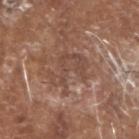- follow-up: total-body-photography surveillance lesion; no biopsy
- lighting: white-light
- lesion diameter: about 5 mm
- site: the head or neck
- patient: male, aged 78 to 82
- TBP lesion metrics: a border-irregularity rating of about 9/10, a within-lesion color-variation index near 2.5/10, and radial color variation of about 1; an automated nevus-likeness rating near 0 out of 100 and lesion-presence confidence of about 80/100
- acquisition: ~15 mm tile from a whole-body skin photo